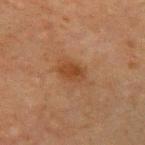biopsy status — imaged on a skin check; not biopsied
subject — male, aged approximately 65
site — the right upper arm
image — total-body-photography crop, ~15 mm field of view